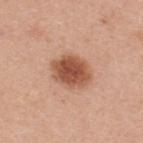Q: Is there a histopathology result?
A: total-body-photography surveillance lesion; no biopsy
Q: Patient demographics?
A: female, aged 28–32
Q: How large is the lesion?
A: ~4.5 mm (longest diameter)
Q: How was this image acquired?
A: 15 mm crop, total-body photography
Q: Lesion location?
A: the upper back